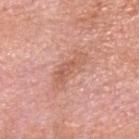{
  "biopsy_status": "not biopsied; imaged during a skin examination",
  "lighting": "white-light",
  "patient": {
    "sex": "male",
    "age_approx": 80
  },
  "site": "head or neck",
  "image": {
    "source": "total-body photography crop",
    "field_of_view_mm": 15
  },
  "lesion_size": {
    "long_diameter_mm_approx": 5.0
  }
}